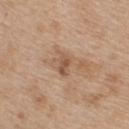Captured during whole-body skin photography for melanoma surveillance; the lesion was not biopsied.
A roughly 15 mm field-of-view crop from a total-body skin photograph.
Captured under white-light illumination.
Located on the upper back.
About 2.5 mm across.
A female patient, in their mid- to late 40s.